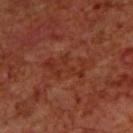{
  "biopsy_status": "not biopsied; imaged during a skin examination",
  "image": {
    "source": "total-body photography crop",
    "field_of_view_mm": 15
  },
  "patient": {
    "sex": "male",
    "age_approx": 70
  },
  "automated_metrics": {
    "area_mm2_approx": 9.0,
    "shape_asymmetry": 0.55,
    "border_irregularity_0_10": 9.0,
    "color_variation_0_10": 2.0,
    "peripheral_color_asymmetry": 0.5,
    "nevus_likeness_0_100": 0,
    "lesion_detection_confidence_0_100": 85
  },
  "lesion_size": {
    "long_diameter_mm_approx": 5.5
  },
  "site": "upper back",
  "lighting": "cross-polarized"
}